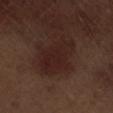notes — no biopsy performed (imaged during a skin exam).